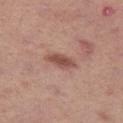Imaged during a routine full-body skin examination; the lesion was not biopsied and no histopathology is available.
The tile uses white-light illumination.
The patient is a female aged around 40.
A lesion tile, about 15 mm wide, cut from a 3D total-body photograph.
The lesion is on the left thigh.
The lesion's longest dimension is about 4 mm.